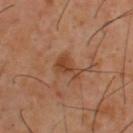| feature | finding |
|---|---|
| biopsy status | total-body-photography surveillance lesion; no biopsy |
| image source | total-body-photography crop, ~15 mm field of view |
| anatomic site | the upper back |
| subject | male, about 40 years old |